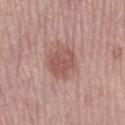Clinical impression: Captured during whole-body skin photography for melanoma surveillance; the lesion was not biopsied. Background: On the left lower leg. Cropped from a whole-body photographic skin survey; the tile spans about 15 mm. A female patient about 65 years old.